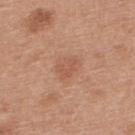Impression: This lesion was catalogued during total-body skin photography and was not selected for biopsy. Background: A male patient about 30 years old. Imaged with white-light lighting. The total-body-photography lesion software estimated a footprint of about 4.5 mm², an eccentricity of roughly 0.75, and two-axis asymmetry of about 0.3. The analysis additionally found an average lesion color of about L≈55 a*≈23 b*≈31 (CIELAB), a lesion–skin lightness drop of about 6, and a normalized lesion–skin contrast near 4.5. From the upper back. A region of skin cropped from a whole-body photographic capture, roughly 15 mm wide.